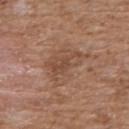Recorded during total-body skin imaging; not selected for excision or biopsy. The lesion is located on the chest. A 15 mm close-up tile from a total-body photography series done for melanoma screening. The lesion's longest dimension is about 5.5 mm. An algorithmic analysis of the crop reported a footprint of about 14 mm², an outline eccentricity of about 0.7 (0 = round, 1 = elongated), and two-axis asymmetry of about 0.35. It also reported a border-irregularity index near 4.5/10 and a within-lesion color-variation index near 3.5/10. And it measured a nevus-likeness score of about 0/100. A male subject, in their 60s.